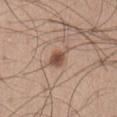The lesion was tiled from a total-body skin photograph and was not biopsied.
Cropped from a whole-body photographic skin survey; the tile spans about 15 mm.
The lesion is on the abdomen.
A male subject approximately 65 years of age.
Automated image analysis of the tile measured a lesion color around L≈51 a*≈19 b*≈28 in CIELAB and roughly 12 lightness units darker than nearby skin. And it measured an automated nevus-likeness rating near 95 out of 100 and a detector confidence of about 100 out of 100 that the crop contains a lesion.
Measured at roughly 3 mm in maximum diameter.